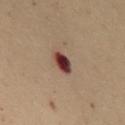<lesion>
<biopsy_status>not biopsied; imaged during a skin examination</biopsy_status>
<site>chest</site>
<automated_metrics>
  <eccentricity>0.65</eccentricity>
  <shape_asymmetry>0.25</shape_asymmetry>
  <nevus_likeness_0_100>0</nevus_likeness_0_100>
</automated_metrics>
<image>
  <source>total-body photography crop</source>
  <field_of_view_mm>15</field_of_view_mm>
</image>
<lesion_size>
  <long_diameter_mm_approx>2.5</long_diameter_mm_approx>
</lesion_size>
<patient>
  <sex>female</sex>
  <age_approx>50</age_approx>
</patient>
<lighting>cross-polarized</lighting>
</lesion>